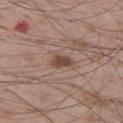Impression:
Captured during whole-body skin photography for melanoma surveillance; the lesion was not biopsied.
Context:
A male patient, aged approximately 50. A roughly 15 mm field-of-view crop from a total-body skin photograph. The total-body-photography lesion software estimated a shape eccentricity near 0.75. The analysis additionally found a lesion color around L≈46 a*≈18 b*≈24 in CIELAB, about 11 CIELAB-L* units darker than the surrounding skin, and a lesion-to-skin contrast of about 8.5 (normalized; higher = more distinct). The software also gave a border-irregularity index near 3.5/10, a color-variation rating of about 2.5/10, and radial color variation of about 1. On the left thigh. This is a white-light tile. Measured at roughly 3 mm in maximum diameter.